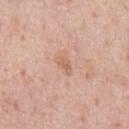biopsy status — total-body-photography surveillance lesion; no biopsy
image source — ~15 mm tile from a whole-body skin photo
patient — male, aged 38–42
location — the chest
lighting — white-light
lesion size — ~2.5 mm (longest diameter)